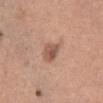Assessment:
No biopsy was performed on this lesion — it was imaged during a full skin examination and was not determined to be concerning.
Image and clinical context:
A close-up tile cropped from a whole-body skin photograph, about 15 mm across. From the abdomen. The tile uses white-light illumination. A female subject, aged approximately 50. Approximately 3.5 mm at its widest. The total-body-photography lesion software estimated an eccentricity of roughly 0.8 and a shape-asymmetry score of about 0.3 (0 = symmetric). It also reported a lesion color around L≈55 a*≈21 b*≈28 in CIELAB, about 11 CIELAB-L* units darker than the surrounding skin, and a lesion-to-skin contrast of about 7.5 (normalized; higher = more distinct).